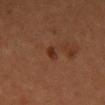  biopsy_status: not biopsied; imaged during a skin examination
  patient:
    sex: female
    age_approx: 65
  image:
    source: total-body photography crop
    field_of_view_mm: 15
  site: chest